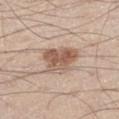<record>
<lesion_size>
  <long_diameter_mm_approx>4.5</long_diameter_mm_approx>
</lesion_size>
<site>leg</site>
<patient>
  <sex>male</sex>
  <age_approx>60</age_approx>
</patient>
<automated_metrics>
  <cielab_L>57</cielab_L>
  <cielab_a>18</cielab_a>
  <cielab_b>28</cielab_b>
  <vs_skin_darker_L>13.0</vs_skin_darker_L>
  <vs_skin_contrast_norm>8.5</vs_skin_contrast_norm>
  <border_irregularity_0_10>3.5</border_irregularity_0_10>
  <color_variation_0_10>6.0</color_variation_0_10>
  <peripheral_color_asymmetry>2.0</peripheral_color_asymmetry>
  <lesion_detection_confidence_0_100>100</lesion_detection_confidence_0_100>
</automated_metrics>
<image>
  <source>total-body photography crop</source>
  <field_of_view_mm>15</field_of_view_mm>
</image>
<lighting>white-light</lighting>
</record>